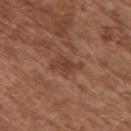Part of a total-body skin-imaging series; this lesion was reviewed on a skin check and was not flagged for biopsy.
The lesion is on the upper back.
The tile uses white-light illumination.
A male patient, in their mid- to late 70s.
Measured at roughly 4 mm in maximum diameter.
Automated tile analysis of the lesion measured an area of roughly 6.5 mm², an outline eccentricity of about 0.85 (0 = round, 1 = elongated), and a shape-asymmetry score of about 0.35 (0 = symmetric). And it measured border irregularity of about 3.5 on a 0–10 scale, a color-variation rating of about 2/10, and radial color variation of about 0.5. The analysis additionally found a nevus-likeness score of about 0/100 and a lesion-detection confidence of about 100/100.
A 15 mm close-up tile from a total-body photography series done for melanoma screening.